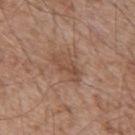The lesion was tiled from a total-body skin photograph and was not biopsied.
This is a white-light tile.
The total-body-photography lesion software estimated a lesion area of about 6 mm², a shape eccentricity near 0.9, and two-axis asymmetry of about 0.35. And it measured a border-irregularity index near 4/10.
Located on the mid back.
A male patient in their mid-50s.
A close-up tile cropped from a whole-body skin photograph, about 15 mm across.
About 4.5 mm across.